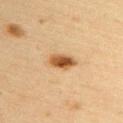About 3.5 mm across. From the upper back. A female subject approximately 45 years of age. Cropped from a total-body skin-imaging series; the visible field is about 15 mm.